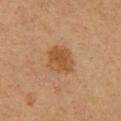Q: Was this lesion biopsied?
A: catalogued during a skin exam; not biopsied
Q: Who is the patient?
A: male, aged 58 to 62
Q: Automated lesion metrics?
A: a footprint of about 8.5 mm², a shape eccentricity near 0.55, and two-axis asymmetry of about 0.15; an average lesion color of about L≈44 a*≈19 b*≈35 (CIELAB) and a normalized border contrast of about 7.5; a nevus-likeness score of about 80/100 and a lesion-detection confidence of about 100/100
Q: How large is the lesion?
A: about 3.5 mm
Q: How was this image acquired?
A: ~15 mm tile from a whole-body skin photo